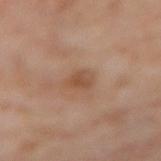Q: Is there a histopathology result?
A: imaged on a skin check; not biopsied
Q: Lesion size?
A: ~2.5 mm (longest diameter)
Q: Illumination type?
A: cross-polarized
Q: Automated lesion metrics?
A: about 8 CIELAB-L* units darker than the surrounding skin
Q: Patient demographics?
A: female, approximately 55 years of age
Q: How was this image acquired?
A: total-body-photography crop, ~15 mm field of view
Q: Lesion location?
A: the right thigh